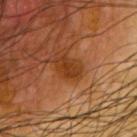Clinical impression:
This lesion was catalogued during total-body skin photography and was not selected for biopsy.
Background:
The recorded lesion diameter is about 2.5 mm. This image is a 15 mm lesion crop taken from a total-body photograph. A male subject, aged 63 to 67. From the chest. Captured under cross-polarized illumination. Automated image analysis of the tile measured a mean CIELAB color near L≈38 a*≈27 b*≈40, roughly 9 lightness units darker than nearby skin, and a lesion-to-skin contrast of about 8 (normalized; higher = more distinct). It also reported a border-irregularity index near 4/10, a within-lesion color-variation index near 1/10, and peripheral color asymmetry of about 0.5.